The lesion's longest dimension is about 6 mm; The total-body-photography lesion software estimated a mean CIELAB color near L≈35 a*≈21 b*≈30 and about 13 CIELAB-L* units darker than the surrounding skin. It also reported a border-irregularity rating of about 3/10, a color-variation rating of about 4.5/10, and radial color variation of about 1; a lesion tile, about 15 mm wide, cut from a 3D total-body photograph; from the chest; the patient is a male roughly 50 years of age; the tile uses cross-polarized illumination:
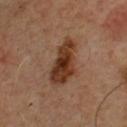Case summary:
* biopsy diagnosis · an atypical melanocytic neoplasm (borderline)